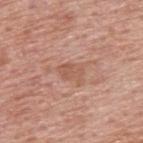follow-up: catalogued during a skin exam; not biopsied
lighting: white-light
diameter: ~3 mm (longest diameter)
acquisition: ~15 mm crop, total-body skin-cancer survey
TBP lesion metrics: a lesion area of about 5 mm², an eccentricity of roughly 0.75, and a shape-asymmetry score of about 0.35 (0 = symmetric); a mean CIELAB color near L≈56 a*≈23 b*≈29, about 7 CIELAB-L* units darker than the surrounding skin, and a lesion-to-skin contrast of about 5.5 (normalized; higher = more distinct); a border-irregularity rating of about 3.5/10, internal color variation of about 1.5 on a 0–10 scale, and a peripheral color-asymmetry measure near 0.5
site: the upper back
patient: male, aged around 75